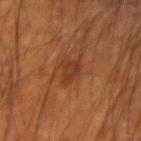Clinical impression: This lesion was catalogued during total-body skin photography and was not selected for biopsy. Context: A close-up tile cropped from a whole-body skin photograph, about 15 mm across. A male subject in their mid-50s. Longest diameter approximately 3 mm. On the left forearm. This is a cross-polarized tile.